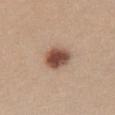Notes:
- biopsy status — catalogued during a skin exam; not biopsied